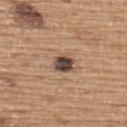{
  "biopsy_status": "not biopsied; imaged during a skin examination",
  "automated_metrics": {
    "area_mm2_approx": 5.0,
    "eccentricity": 0.45,
    "shape_asymmetry": 0.15,
    "cielab_L": 45,
    "cielab_a": 16,
    "cielab_b": 23,
    "vs_skin_darker_L": 17.0,
    "vs_skin_contrast_norm": 13.5,
    "border_irregularity_0_10": 1.5,
    "peripheral_color_asymmetry": 1.5
  },
  "patient": {
    "sex": "male",
    "age_approx": 65
  },
  "lighting": "white-light",
  "lesion_size": {
    "long_diameter_mm_approx": 2.5
  },
  "image": {
    "source": "total-body photography crop",
    "field_of_view_mm": 15
  },
  "site": "upper back"
}